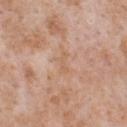Impression: Captured during whole-body skin photography for melanoma surveillance; the lesion was not biopsied. Background: From the chest. A 15 mm crop from a total-body photograph taken for skin-cancer surveillance. The patient is a male roughly 65 years of age. Imaged with white-light lighting. Approximately 2.5 mm at its widest. Automated tile analysis of the lesion measured an area of roughly 2.5 mm², an outline eccentricity of about 0.9 (0 = round, 1 = elongated), and a shape-asymmetry score of about 0.45 (0 = symmetric). The software also gave border irregularity of about 5 on a 0–10 scale and a within-lesion color-variation index near 0/10.